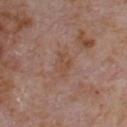• follow-up · total-body-photography surveillance lesion; no biopsy
• TBP lesion metrics · a lesion color around L≈48 a*≈20 b*≈28 in CIELAB, roughly 5 lightness units darker than nearby skin, and a lesion-to-skin contrast of about 5.5 (normalized; higher = more distinct); border irregularity of about 2.5 on a 0–10 scale, a color-variation rating of about 2/10, and radial color variation of about 0.5
• diameter · about 3 mm
• image source · total-body-photography crop, ~15 mm field of view
• location · the chest
• patient · male, aged approximately 65